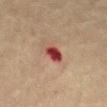notes: no biopsy performed (imaged during a skin exam)
subject: male, in their mid-70s
anatomic site: the mid back
acquisition: 15 mm crop, total-body photography
diameter: ~2.5 mm (longest diameter)
illumination: cross-polarized
automated metrics: a border-irregularity index near 1.5/10, internal color variation of about 2.5 on a 0–10 scale, and peripheral color asymmetry of about 0.5; a classifier nevus-likeness of about 0/100